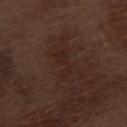The lesion was tiled from a total-body skin photograph and was not biopsied.
A male patient, aged around 70.
The lesion is located on the left thigh.
Measured at roughly 4 mm in maximum diameter.
A close-up tile cropped from a whole-body skin photograph, about 15 mm across.
Imaged with white-light lighting.